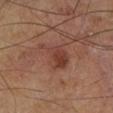workup = imaged on a skin check; not biopsied
site = the right lower leg
automated metrics = an average lesion color of about L≈40 a*≈23 b*≈27 (CIELAB) and about 8 CIELAB-L* units darker than the surrounding skin; a lesion-detection confidence of about 100/100
lesion size = about 5 mm
image = 15 mm crop, total-body photography
patient = male, roughly 65 years of age
illumination = cross-polarized